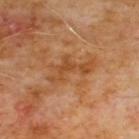Clinical impression: This lesion was catalogued during total-body skin photography and was not selected for biopsy. Context: The patient is a male aged 58 to 62. The lesion is on the front of the torso. The tile uses cross-polarized illumination. Approximately 5.5 mm at its widest. Cropped from a whole-body photographic skin survey; the tile spans about 15 mm.